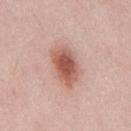workup = imaged on a skin check; not biopsied | image = 15 mm crop, total-body photography | lighting = white-light illumination | lesion size = ~4.5 mm (longest diameter) | TBP lesion metrics = a border-irregularity rating of about 2/10, a within-lesion color-variation index near 5.5/10, and peripheral color asymmetry of about 1.5 | subject = male, approximately 50 years of age | anatomic site = the back.